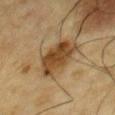<case>
  <lighting>cross-polarized</lighting>
  <patient>
    <sex>male</sex>
    <age_approx>85+</age_approx>
  </patient>
  <image>
    <source>total-body photography crop</source>
    <field_of_view_mm>15</field_of_view_mm>
  </image>
  <lesion_size>
    <long_diameter_mm_approx>5.0</long_diameter_mm_approx>
  </lesion_size>
  <site>chest</site>
  <automated_metrics>
    <area_mm2_approx>14.0</area_mm2_approx>
    <eccentricity>0.6</eccentricity>
    <shape_asymmetry>0.2</shape_asymmetry>
    <border_irregularity_0_10>2.5</border_irregularity_0_10>
    <color_variation_0_10>4.5</color_variation_0_10>
    <peripheral_color_asymmetry>1.5</peripheral_color_asymmetry>
    <nevus_likeness_0_100>80</nevus_likeness_0_100>
    <lesion_detection_confidence_0_100>100</lesion_detection_confidence_0_100>
  </automated_metrics>
</case>